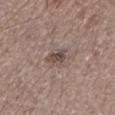No biopsy was performed on this lesion — it was imaged during a full skin examination and was not determined to be concerning. A 15 mm crop from a total-body photograph taken for skin-cancer surveillance. A male subject, in their mid- to late 60s. The lesion's longest dimension is about 2.5 mm. The lesion is located on the left lower leg. This is a white-light tile.